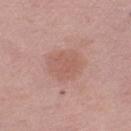Recorded during total-body skin imaging; not selected for excision or biopsy. The subject is a female aged around 65. A roughly 15 mm field-of-view crop from a total-body skin photograph. Located on the left thigh.